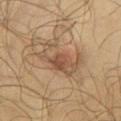<case>
  <biopsy_status>not biopsied; imaged during a skin examination</biopsy_status>
  <lighting>cross-polarized</lighting>
  <image>
    <source>total-body photography crop</source>
    <field_of_view_mm>15</field_of_view_mm>
  </image>
  <site>chest</site>
  <patient>
    <sex>male</sex>
    <age_approx>65</age_approx>
  </patient>
  <automated_metrics>
    <area_mm2_approx>12.0</area_mm2_approx>
    <eccentricity>0.8</eccentricity>
    <shape_asymmetry>0.55</shape_asymmetry>
    <cielab_L>48</cielab_L>
    <cielab_a>16</cielab_a>
    <cielab_b>30</cielab_b>
    <vs_skin_darker_L>9.0</vs_skin_darker_L>
    <vs_skin_contrast_norm>7.0</vs_skin_contrast_norm>
  </automated_metrics>
</case>